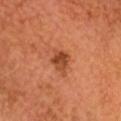Q: Was this lesion biopsied?
A: imaged on a skin check; not biopsied
Q: What lighting was used for the tile?
A: cross-polarized
Q: What kind of image is this?
A: 15 mm crop, total-body photography
Q: Lesion size?
A: about 3 mm
Q: Patient demographics?
A: male, in their mid-60s